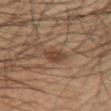  biopsy_status: not biopsied; imaged during a skin examination
  automated_metrics:
    area_mm2_approx: 5.0
    shape_asymmetry: 0.3
    cielab_L: 38
    cielab_a: 16
    cielab_b: 26
    vs_skin_contrast_norm: 7.0
  lesion_size:
    long_diameter_mm_approx: 3.0
  image:
    source: total-body photography crop
    field_of_view_mm: 15
  site: right forearm
  patient:
    sex: male
    age_approx: 60